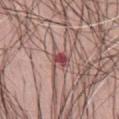notes=imaged on a skin check; not biopsied | lighting=white-light | automated lesion analysis=a normalized border contrast of about 8.5; a classifier nevus-likeness of about 5/100 and a lesion-detection confidence of about 100/100 | site=the abdomen | lesion diameter=~3 mm (longest diameter) | patient=female, aged around 60 | acquisition=total-body-photography crop, ~15 mm field of view.